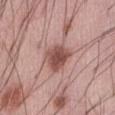Q: Is there a histopathology result?
A: imaged on a skin check; not biopsied
Q: What are the patient's age and sex?
A: male, approximately 55 years of age
Q: What is the lesion's diameter?
A: about 3.5 mm
Q: Illumination type?
A: white-light illumination
Q: What is the anatomic site?
A: the abdomen
Q: How was this image acquired?
A: 15 mm crop, total-body photography